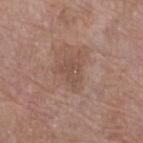Clinical impression:
Captured during whole-body skin photography for melanoma surveillance; the lesion was not biopsied.
Acquisition and patient details:
The lesion is on the left lower leg. The patient is a female aged around 70. A close-up tile cropped from a whole-body skin photograph, about 15 mm across. This is a white-light tile.